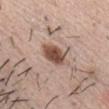This lesion was catalogued during total-body skin photography and was not selected for biopsy. The recorded lesion diameter is about 4 mm. A male subject, in their mid-30s. A region of skin cropped from a whole-body photographic capture, roughly 15 mm wide. The lesion is on the chest.